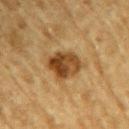{
  "biopsy_status": "not biopsied; imaged during a skin examination",
  "lighting": "cross-polarized",
  "site": "right upper arm",
  "lesion_size": {
    "long_diameter_mm_approx": 4.0
  },
  "image": {
    "source": "total-body photography crop",
    "field_of_view_mm": 15
  },
  "patient": {
    "sex": "male",
    "age_approx": "85+"
  }
}